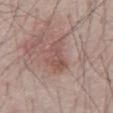This lesion was catalogued during total-body skin photography and was not selected for biopsy.
Approximately 4.5 mm at its widest.
The patient is a male aged 63 to 67.
The tile uses white-light illumination.
Cropped from a total-body skin-imaging series; the visible field is about 15 mm.
On the abdomen.